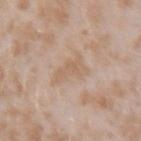Image and clinical context: Longest diameter approximately 4.5 mm. Imaged with white-light lighting. A female subject, aged 23 to 27. Automated tile analysis of the lesion measured an area of roughly 8 mm², an eccentricity of roughly 0.9, and a shape-asymmetry score of about 0.45 (0 = symmetric). The software also gave an average lesion color of about L≈62 a*≈16 b*≈30 (CIELAB) and a lesion–skin lightness drop of about 6. The lesion is located on the arm. A 15 mm close-up extracted from a 3D total-body photography capture.